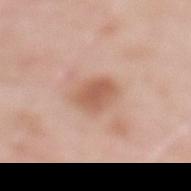Q: Was a biopsy performed?
A: catalogued during a skin exam; not biopsied
Q: Patient demographics?
A: female, aged 48–52
Q: What kind of image is this?
A: ~15 mm tile from a whole-body skin photo
Q: What is the lesion's diameter?
A: ~3.5 mm (longest diameter)
Q: How was the tile lit?
A: white-light illumination
Q: Automated lesion metrics?
A: a mean CIELAB color near L≈59 a*≈22 b*≈31, about 10 CIELAB-L* units darker than the surrounding skin, and a normalized lesion–skin contrast near 7.5
Q: Lesion location?
A: the upper back